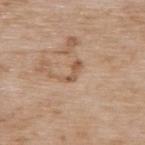The lesion was photographed on a routine skin check and not biopsied; there is no pathology result. Automated image analysis of the tile measured an area of roughly 3.5 mm², an eccentricity of roughly 0.9, and a symmetry-axis asymmetry near 0.5. The patient is a female approximately 75 years of age. Captured under white-light illumination. Located on the upper back. A lesion tile, about 15 mm wide, cut from a 3D total-body photograph. About 3 mm across.